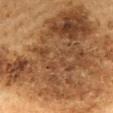Context:
The total-body-photography lesion software estimated a footprint of about 150 mm² and a symmetry-axis asymmetry near 0.45. The analysis additionally found a nevus-likeness score of about 0/100 and a detector confidence of about 80 out of 100 that the crop contains a lesion. The recorded lesion diameter is about 21.5 mm. On the mid back. A male subject, in their 60s. Captured under cross-polarized illumination. A roughly 15 mm field-of-view crop from a total-body skin photograph.